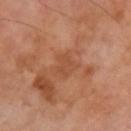Clinical impression:
Captured during whole-body skin photography for melanoma surveillance; the lesion was not biopsied.
Acquisition and patient details:
The lesion is located on the left upper arm. A lesion tile, about 15 mm wide, cut from a 3D total-body photograph. A male patient, in their 70s.